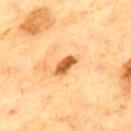Assessment: Captured during whole-body skin photography for melanoma surveillance; the lesion was not biopsied. Clinical summary: Measured at roughly 3 mm in maximum diameter. From the upper back. A region of skin cropped from a whole-body photographic capture, roughly 15 mm wide. A male subject, in their 70s. The lesion-visualizer software estimated an area of roughly 4.5 mm², an outline eccentricity of about 0.9 (0 = round, 1 = elongated), and two-axis asymmetry of about 0.3. And it measured peripheral color asymmetry of about 1. This is a cross-polarized tile.